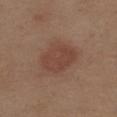<case>
<biopsy_status>not biopsied; imaged during a skin examination</biopsy_status>
<site>upper back</site>
<patient>
  <sex>male</sex>
  <age_approx>70</age_approx>
</patient>
<image>
  <source>total-body photography crop</source>
  <field_of_view_mm>15</field_of_view_mm>
</image>
<lighting>white-light</lighting>
<lesion_size>
  <long_diameter_mm_approx>5.0</long_diameter_mm_approx>
</lesion_size>
</case>